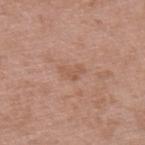Clinical impression:
Captured during whole-body skin photography for melanoma surveillance; the lesion was not biopsied.
Background:
Cropped from a total-body skin-imaging series; the visible field is about 15 mm. Imaged with white-light lighting. A male patient in their 40s. Longest diameter approximately 3 mm. An algorithmic analysis of the crop reported a footprint of about 4 mm², an eccentricity of roughly 0.8, and two-axis asymmetry of about 0.4. The analysis additionally found a mean CIELAB color near L≈55 a*≈21 b*≈30, about 6 CIELAB-L* units darker than the surrounding skin, and a normalized border contrast of about 4.5. From the upper back.